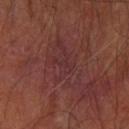* workup — no biopsy performed (imaged during a skin exam)
* illumination — cross-polarized
* subject — male, in their mid- to late 60s
* lesion diameter — ~6 mm (longest diameter)
* site — the left forearm
* imaging modality — ~15 mm tile from a whole-body skin photo
* automated metrics — an area of roughly 14 mm², an outline eccentricity of about 0.85 (0 = round, 1 = elongated), and a shape-asymmetry score of about 0.45 (0 = symmetric); border irregularity of about 7.5 on a 0–10 scale and radial color variation of about 1; a nevus-likeness score of about 0/100 and lesion-presence confidence of about 85/100